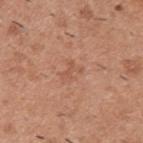Notes:
• notes · catalogued during a skin exam; not biopsied
• subject · male, aged 38 to 42
• diameter · about 2.5 mm
• lighting · white-light illumination
• image source · ~15 mm tile from a whole-body skin photo
• anatomic site · the upper back